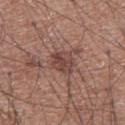<tbp_lesion>
  <biopsy_status>not biopsied; imaged during a skin examination</biopsy_status>
  <patient>
    <sex>male</sex>
    <age_approx>75</age_approx>
  </patient>
  <site>abdomen</site>
  <lesion_size>
    <long_diameter_mm_approx>3.0</long_diameter_mm_approx>
  </lesion_size>
  <image>
    <source>total-body photography crop</source>
    <field_of_view_mm>15</field_of_view_mm>
  </image>
  <lighting>white-light</lighting>
</tbp_lesion>